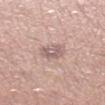Imaged during a routine full-body skin examination; the lesion was not biopsied and no histopathology is available. A 15 mm close-up tile from a total-body photography series done for melanoma screening. A male patient in their 40s. Imaged with white-light lighting. The recorded lesion diameter is about 3 mm. From the right lower leg.